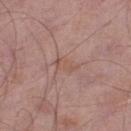Clinical impression: The lesion was tiled from a total-body skin photograph and was not biopsied. Image and clinical context: An algorithmic analysis of the crop reported an outline eccentricity of about 0.9 (0 = round, 1 = elongated) and a symmetry-axis asymmetry near 0.5. The analysis additionally found an average lesion color of about L≈53 a*≈20 b*≈26 (CIELAB), a lesion–skin lightness drop of about 6, and a normalized lesion–skin contrast near 5. The software also gave a classifier nevus-likeness of about 0/100 and a detector confidence of about 95 out of 100 that the crop contains a lesion. A close-up tile cropped from a whole-body skin photograph, about 15 mm across. The patient is a male in their mid-50s. The tile uses white-light illumination. The lesion is on the left lower leg. The recorded lesion diameter is about 3 mm.